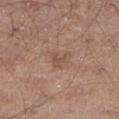Assessment:
Captured during whole-body skin photography for melanoma surveillance; the lesion was not biopsied.
Clinical summary:
A male patient, approximately 55 years of age. Located on the left thigh. A 15 mm crop from a total-body photograph taken for skin-cancer surveillance.